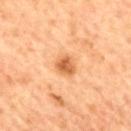biopsy status: total-body-photography surveillance lesion; no biopsy
lesion size: about 2.5 mm
illumination: cross-polarized illumination
subject: male, aged 58 to 62
image: ~15 mm crop, total-body skin-cancer survey
site: the mid back